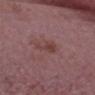Clinical impression: The lesion was photographed on a routine skin check and not biopsied; there is no pathology result. Context: Automated tile analysis of the lesion measured a border-irregularity index near 6/10, internal color variation of about 1.5 on a 0–10 scale, and a peripheral color-asymmetry measure near 0.5. The lesion is on the head or neck. A region of skin cropped from a whole-body photographic capture, roughly 15 mm wide. Approximately 4 mm at its widest. The subject is a male aged 63–67. Captured under white-light illumination.